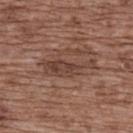Captured during whole-body skin photography for melanoma surveillance; the lesion was not biopsied. The subject is a female about 75 years old. Captured under white-light illumination. Measured at roughly 6.5 mm in maximum diameter. The lesion is on the upper back. A 15 mm crop from a total-body photograph taken for skin-cancer surveillance.